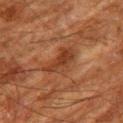follow-up — imaged on a skin check; not biopsied
lighting — cross-polarized illumination
body site — the leg
patient — male, aged around 80
TBP lesion metrics — an average lesion color of about L≈31 a*≈20 b*≈28 (CIELAB), about 7 CIELAB-L* units darker than the surrounding skin, and a normalized lesion–skin contrast near 7; a classifier nevus-likeness of about 0/100 and a detector confidence of about 90 out of 100 that the crop contains a lesion
acquisition — 15 mm crop, total-body photography
lesion size — about 4.5 mm